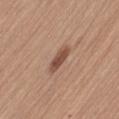tile lighting=white-light
TBP lesion metrics=a border-irregularity index near 2/10
body site=the left thigh
acquisition=total-body-photography crop, ~15 mm field of view
patient=female, aged 63 to 67